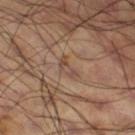Q: Was this lesion biopsied?
A: catalogued during a skin exam; not biopsied
Q: What is the lesion's diameter?
A: ~2.5 mm (longest diameter)
Q: What is the imaging modality?
A: ~15 mm crop, total-body skin-cancer survey
Q: What is the anatomic site?
A: the right thigh
Q: Who is the patient?
A: male, approximately 60 years of age
Q: Illumination type?
A: cross-polarized illumination